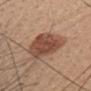Q: Was a biopsy performed?
A: total-body-photography surveillance lesion; no biopsy
Q: What is the imaging modality?
A: 15 mm crop, total-body photography
Q: Who is the patient?
A: female, in their mid- to late 40s
Q: Where on the body is the lesion?
A: the head or neck